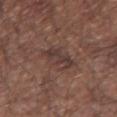Clinical impression:
Imaged during a routine full-body skin examination; the lesion was not biopsied and no histopathology is available.
Image and clinical context:
A 15 mm crop from a total-body photograph taken for skin-cancer surveillance. The subject is a male aged approximately 65. From the left upper arm.